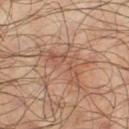notes: catalogued during a skin exam; not biopsied
patient: male, approximately 65 years of age
lesion size: ~5.5 mm (longest diameter)
location: the leg
imaging modality: 15 mm crop, total-body photography
TBP lesion metrics: an outline eccentricity of about 0.9 (0 = round, 1 = elongated) and a symmetry-axis asymmetry near 0.65; an average lesion color of about L≈49 a*≈20 b*≈28 (CIELAB), a lesion–skin lightness drop of about 7, and a normalized lesion–skin contrast near 5.5; a classifier nevus-likeness of about 0/100 and a detector confidence of about 60 out of 100 that the crop contains a lesion